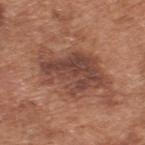biopsy status: no biopsy performed (imaged during a skin exam)
imaging modality: ~15 mm tile from a whole-body skin photo
anatomic site: the upper back
illumination: white-light
patient: male, in their mid-60s